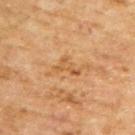Findings:
– notes · catalogued during a skin exam; not biopsied
– tile lighting · cross-polarized illumination
– site · the upper back
– subject · male, aged approximately 65
– lesion diameter · ~3 mm (longest diameter)
– image source · 15 mm crop, total-body photography